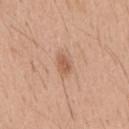Imaged during a routine full-body skin examination; the lesion was not biopsied and no histopathology is available.